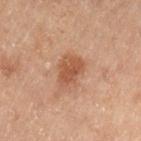Clinical impression: Imaged during a routine full-body skin examination; the lesion was not biopsied and no histopathology is available. Clinical summary: On the left thigh. A close-up tile cropped from a whole-body skin photograph, about 15 mm across. A male patient, aged 68 to 72.